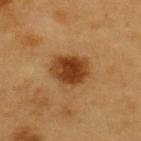Captured during whole-body skin photography for melanoma surveillance; the lesion was not biopsied.
On the upper back.
The lesion's longest dimension is about 4 mm.
This is a cross-polarized tile.
A male subject, about 60 years old.
The lesion-visualizer software estimated a lesion color around L≈41 a*≈24 b*≈39 in CIELAB and roughly 14 lightness units darker than nearby skin. The analysis additionally found a classifier nevus-likeness of about 100/100 and a lesion-detection confidence of about 100/100.
A region of skin cropped from a whole-body photographic capture, roughly 15 mm wide.